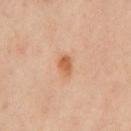Clinical impression: Imaged during a routine full-body skin examination; the lesion was not biopsied and no histopathology is available. Context: A roughly 15 mm field-of-view crop from a total-body skin photograph. From the chest. The patient is a male aged 63–67. Automated tile analysis of the lesion measured an average lesion color of about L≈60 a*≈25 b*≈38 (CIELAB) and a normalized border contrast of about 8. And it measured a border-irregularity index near 2/10 and a color-variation rating of about 2.5/10.